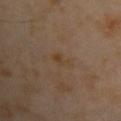Acquisition and patient details:
A roughly 15 mm field-of-view crop from a total-body skin photograph. Measured at roughly 2.5 mm in maximum diameter. Captured under cross-polarized illumination. A female subject, approximately 40 years of age. From the upper back.